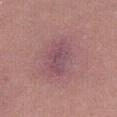<record>
  <biopsy_status>not biopsied; imaged during a skin examination</biopsy_status>
  <lighting>white-light</lighting>
  <image>
    <source>total-body photography crop</source>
    <field_of_view_mm>15</field_of_view_mm>
  </image>
  <patient>
    <sex>female</sex>
    <age_approx>35</age_approx>
  </patient>
  <lesion_size>
    <long_diameter_mm_approx>5.0</long_diameter_mm_approx>
  </lesion_size>
  <automated_metrics>
    <area_mm2_approx>11.0</area_mm2_approx>
    <eccentricity>0.75</eccentricity>
    <shape_asymmetry>0.25</shape_asymmetry>
    <border_irregularity_0_10>3.5</border_irregularity_0_10>
  </automated_metrics>
  <site>left leg</site>
</record>